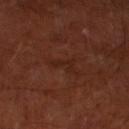Findings:
- follow-up · imaged on a skin check; not biopsied
- body site · the leg
- subject · male, aged around 65
- imaging modality · 15 mm crop, total-body photography